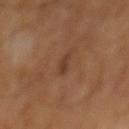Part of a total-body skin-imaging series; this lesion was reviewed on a skin check and was not flagged for biopsy. Captured under cross-polarized illumination. A 15 mm close-up extracted from a 3D total-body photography capture. A male subject, in their mid- to late 60s. The lesion's longest dimension is about 2.5 mm. The lesion-visualizer software estimated an area of roughly 2.5 mm², an outline eccentricity of about 0.9 (0 = round, 1 = elongated), and a symmetry-axis asymmetry near 0.45. The analysis additionally found a lesion color around L≈37 a*≈19 b*≈29 in CIELAB, about 7 CIELAB-L* units darker than the surrounding skin, and a normalized lesion–skin contrast near 6. The software also gave a border-irregularity rating of about 4.5/10 and peripheral color asymmetry of about 0.